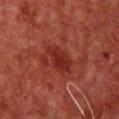Clinical impression: Recorded during total-body skin imaging; not selected for excision or biopsy. Acquisition and patient details: The lesion's longest dimension is about 4 mm. The tile uses cross-polarized illumination. A male patient aged 58 to 62. An algorithmic analysis of the crop reported a border-irregularity rating of about 3/10, a within-lesion color-variation index near 2/10, and peripheral color asymmetry of about 0.5. The analysis additionally found a classifier nevus-likeness of about 10/100 and a detector confidence of about 100 out of 100 that the crop contains a lesion. A 15 mm close-up extracted from a 3D total-body photography capture. Located on the front of the torso.